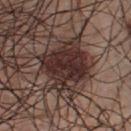workup — total-body-photography surveillance lesion; no biopsy | anatomic site — the front of the torso | subject — male, approximately 35 years of age | image — ~15 mm crop, total-body skin-cancer survey | diameter — about 5 mm | illumination — white-light | automated lesion analysis — a footprint of about 19 mm², a shape eccentricity near 0.3, and a shape-asymmetry score of about 0.25 (0 = symmetric); a lesion color around L≈29 a*≈17 b*≈18 in CIELAB; border irregularity of about 3.5 on a 0–10 scale and a peripheral color-asymmetry measure near 1.5; a nevus-likeness score of about 70/100 and a lesion-detection confidence of about 100/100.